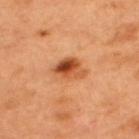Assessment:
The lesion was photographed on a routine skin check and not biopsied; there is no pathology result.
Image and clinical context:
Located on the back. Automated image analysis of the tile measured a lesion color around L≈52 a*≈29 b*≈41 in CIELAB, a lesion–skin lightness drop of about 14, and a lesion-to-skin contrast of about 9.5 (normalized; higher = more distinct). It also reported a classifier nevus-likeness of about 95/100 and a lesion-detection confidence of about 100/100. Imaged with cross-polarized lighting. A 15 mm close-up tile from a total-body photography series done for melanoma screening. Approximately 4 mm at its widest. A male patient approximately 50 years of age.